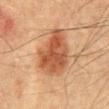biopsy status: no biopsy performed (imaged during a skin exam)
diameter: ~6 mm (longest diameter)
tile lighting: cross-polarized
site: the abdomen
subject: male, approximately 50 years of age
imaging modality: ~15 mm crop, total-body skin-cancer survey